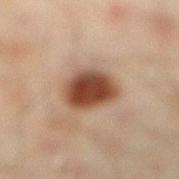| feature | finding |
|---|---|
| notes | catalogued during a skin exam; not biopsied |
| site | the leg |
| image source | ~15 mm crop, total-body skin-cancer survey |
| subject | male, in their mid-40s |